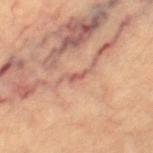Assessment: Imaged during a routine full-body skin examination; the lesion was not biopsied and no histopathology is available. Clinical summary: The total-body-photography lesion software estimated a lesion color around L≈54 a*≈24 b*≈26 in CIELAB, roughly 9 lightness units darker than nearby skin, and a lesion-to-skin contrast of about 6.5 (normalized; higher = more distinct). It also reported internal color variation of about 0 on a 0–10 scale and a peripheral color-asymmetry measure near 0. A region of skin cropped from a whole-body photographic capture, roughly 15 mm wide. Imaged with cross-polarized lighting. The lesion's longest dimension is about 3 mm. On the right thigh. A female patient about 65 years old.